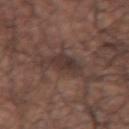Captured during whole-body skin photography for melanoma surveillance; the lesion was not biopsied.
On the left forearm.
A lesion tile, about 15 mm wide, cut from a 3D total-body photograph.
Approximately 4.5 mm at its widest.
Automated tile analysis of the lesion measured a mean CIELAB color near L≈34 a*≈16 b*≈19 and a normalized lesion–skin contrast near 7. It also reported internal color variation of about 4 on a 0–10 scale and peripheral color asymmetry of about 1. And it measured a nevus-likeness score of about 15/100 and lesion-presence confidence of about 90/100.
A male patient aged 63 to 67.